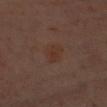{"biopsy_status": "not biopsied; imaged during a skin examination", "site": "left upper arm", "patient": {"sex": "female", "age_approx": 65}, "automated_metrics": {"vs_skin_darker_L": 4.0, "vs_skin_contrast_norm": 5.5}, "image": {"source": "total-body photography crop", "field_of_view_mm": 15}, "lighting": "cross-polarized"}